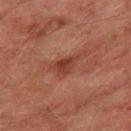A 15 mm close-up tile from a total-body photography series done for melanoma screening. Automated tile analysis of the lesion measured border irregularity of about 4.5 on a 0–10 scale and peripheral color asymmetry of about 1. Imaged with cross-polarized lighting. A male patient aged approximately 75. The lesion is located on the right thigh. About 4 mm across.